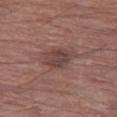notes=no biopsy performed (imaged during a skin exam); imaging modality=~15 mm tile from a whole-body skin photo; location=the left thigh; illumination=white-light illumination; size=≈4 mm; automated lesion analysis=an automated nevus-likeness rating near 15 out of 100 and a lesion-detection confidence of about 100/100; patient=male, aged 73–77.